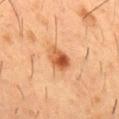Clinical impression: Recorded during total-body skin imaging; not selected for excision or biopsy. Acquisition and patient details: The lesion is located on the chest. A male patient, in their mid-50s. The total-body-photography lesion software estimated a lesion area of about 6.5 mm² and an outline eccentricity of about 0.7 (0 = round, 1 = elongated). It also reported an average lesion color of about L≈50 a*≈24 b*≈37 (CIELAB) and a lesion-to-skin contrast of about 8.5 (normalized; higher = more distinct). The analysis additionally found a nevus-likeness score of about 100/100 and a lesion-detection confidence of about 100/100. Measured at roughly 3.5 mm in maximum diameter. Captured under cross-polarized illumination. A 15 mm crop from a total-body photograph taken for skin-cancer surveillance.